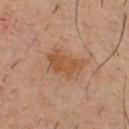site=the chest
subject=male, in their mid-30s
image=total-body-photography crop, ~15 mm field of view
diameter=about 4.5 mm